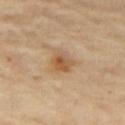notes: no biopsy performed (imaged during a skin exam) | automated metrics: a lesion area of about 4 mm², an eccentricity of roughly 0.6, and two-axis asymmetry of about 0.25; a mean CIELAB color near L≈55 a*≈19 b*≈37 and a lesion-to-skin contrast of about 8 (normalized; higher = more distinct); an automated nevus-likeness rating near 50 out of 100 and a lesion-detection confidence of about 100/100 | illumination: cross-polarized | anatomic site: the right thigh | acquisition: ~15 mm tile from a whole-body skin photo | lesion size: ≈2.5 mm | patient: female, aged 68–72.